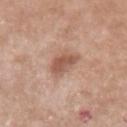follow-up: no biopsy performed (imaged during a skin exam); automated lesion analysis: border irregularity of about 2.5 on a 0–10 scale, a color-variation rating of about 3/10, and radial color variation of about 1; subject: female, aged 53–57; acquisition: 15 mm crop, total-body photography; location: the arm; lesion diameter: ≈3 mm; tile lighting: white-light illumination.